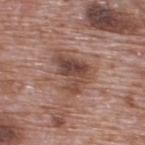Impression:
Captured during whole-body skin photography for melanoma surveillance; the lesion was not biopsied.
Acquisition and patient details:
The lesion is on the upper back. A male patient, aged 68 to 72. This is a white-light tile. About 5 mm across. A 15 mm crop from a total-body photograph taken for skin-cancer surveillance. Automated tile analysis of the lesion measured a lesion area of about 14 mm² and a symmetry-axis asymmetry near 0.45. The software also gave a lesion–skin lightness drop of about 11 and a normalized border contrast of about 8. It also reported a border-irregularity rating of about 5/10, a within-lesion color-variation index near 7/10, and peripheral color asymmetry of about 2.5. It also reported a lesion-detection confidence of about 100/100.